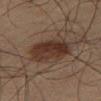The lesion was photographed on a routine skin check and not biopsied; there is no pathology result. Approximately 5 mm at its widest. A male subject, roughly 65 years of age. Cropped from a whole-body photographic skin survey; the tile spans about 15 mm. On the right thigh.